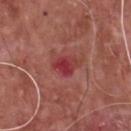Captured during whole-body skin photography for melanoma surveillance; the lesion was not biopsied. Imaged with white-light lighting. Automated tile analysis of the lesion measured a color-variation rating of about 7/10 and a peripheral color-asymmetry measure near 2.5. It also reported a nevus-likeness score of about 0/100 and a detector confidence of about 100 out of 100 that the crop contains a lesion. On the chest. A region of skin cropped from a whole-body photographic capture, roughly 15 mm wide. A male patient aged 63–67. Longest diameter approximately 3.5 mm.